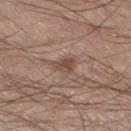Captured during whole-body skin photography for melanoma surveillance; the lesion was not biopsied.
From the left lower leg.
A 15 mm crop from a total-body photograph taken for skin-cancer surveillance.
Longest diameter approximately 2.5 mm.
Imaged with white-light lighting.
Automated image analysis of the tile measured a footprint of about 4 mm², an eccentricity of roughly 0.75, and two-axis asymmetry of about 0.4. And it measured a mean CIELAB color near L≈47 a*≈17 b*≈26, about 9 CIELAB-L* units darker than the surrounding skin, and a normalized border contrast of about 7.
A male patient, aged around 30.